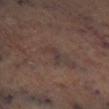Findings:
• workup · catalogued during a skin exam; not biopsied
• lesion diameter · about 3 mm
• anatomic site · the right lower leg
• image source · ~15 mm crop, total-body skin-cancer survey
• tile lighting · cross-polarized illumination
• image-analysis metrics · an area of roughly 3 mm², a shape eccentricity near 0.9, and two-axis asymmetry of about 0.65; a mean CIELAB color near L≈36 a*≈15 b*≈18, a lesion–skin lightness drop of about 5, and a normalized border contrast of about 6; border irregularity of about 7 on a 0–10 scale and radial color variation of about 0; a classifier nevus-likeness of about 0/100 and a detector confidence of about 50 out of 100 that the crop contains a lesion